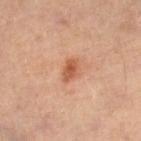Recorded during total-body skin imaging; not selected for excision or biopsy. The lesion is located on the right lower leg. The tile uses cross-polarized illumination. The recorded lesion diameter is about 2.5 mm. A lesion tile, about 15 mm wide, cut from a 3D total-body photograph. A female subject aged 58 to 62.